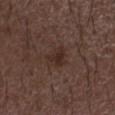Recorded during total-body skin imaging; not selected for excision or biopsy.
The patient is a male about 50 years old.
Captured under white-light illumination.
On the left forearm.
The total-body-photography lesion software estimated an automated nevus-likeness rating near 10 out of 100 and a lesion-detection confidence of about 100/100.
Cropped from a whole-body photographic skin survey; the tile spans about 15 mm.
The lesion's longest dimension is about 3 mm.